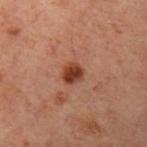Imaged during a routine full-body skin examination; the lesion was not biopsied and no histopathology is available. The lesion is on the left upper arm. The subject is a female aged around 55. This is a cross-polarized tile. A close-up tile cropped from a whole-body skin photograph, about 15 mm across. About 2.5 mm across. The lesion-visualizer software estimated an average lesion color of about L≈33 a*≈23 b*≈27 (CIELAB), a lesion–skin lightness drop of about 12, and a lesion-to-skin contrast of about 10.5 (normalized; higher = more distinct). The software also gave a border-irregularity index near 1.5/10 and a peripheral color-asymmetry measure near 1. The software also gave an automated nevus-likeness rating near 95 out of 100 and a lesion-detection confidence of about 100/100.